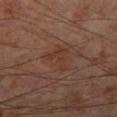follow-up = total-body-photography surveillance lesion; no biopsy
subject = male, aged approximately 55
size = ~3.5 mm (longest diameter)
image source = ~15 mm tile from a whole-body skin photo
location = the right lower leg
lighting = cross-polarized illumination